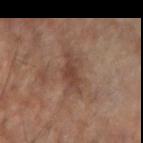This lesion was catalogued during total-body skin photography and was not selected for biopsy.
An algorithmic analysis of the crop reported an outline eccentricity of about 0.85 (0 = round, 1 = elongated) and a symmetry-axis asymmetry near 0.45. It also reported an automated nevus-likeness rating near 0 out of 100 and lesion-presence confidence of about 100/100.
On the arm.
A male subject about 65 years old.
Captured under cross-polarized illumination.
Cropped from a whole-body photographic skin survey; the tile spans about 15 mm.